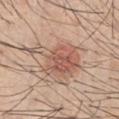biopsy_status: not biopsied; imaged during a skin examination
patient:
  sex: male
  age_approx: 45
image:
  source: total-body photography crop
  field_of_view_mm: 15
site: chest